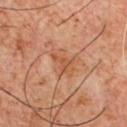Assessment:
Part of a total-body skin-imaging series; this lesion was reviewed on a skin check and was not flagged for biopsy.
Image and clinical context:
Approximately 3 mm at its widest. The total-body-photography lesion software estimated a footprint of about 4.5 mm², an eccentricity of roughly 0.85, and a symmetry-axis asymmetry near 0.5. The analysis additionally found a lesion color around L≈52 a*≈25 b*≈35 in CIELAB, roughly 7 lightness units darker than nearby skin, and a normalized border contrast of about 6. The software also gave a border-irregularity rating of about 5.5/10 and peripheral color asymmetry of about 0.5. The patient is a male roughly 60 years of age. A 15 mm close-up tile from a total-body photography series done for melanoma screening. The lesion is on the front of the torso. The tile uses cross-polarized illumination.